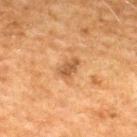size: ~3 mm (longest diameter)
location: the upper back
imaging modality: total-body-photography crop, ~15 mm field of view
patient: male, aged approximately 50
lighting: cross-polarized illumination
automated lesion analysis: an area of roughly 4 mm², a shape eccentricity near 0.85, and two-axis asymmetry of about 0.25; a lesion color around L≈45 a*≈19 b*≈34 in CIELAB and about 9 CIELAB-L* units darker than the surrounding skin; border irregularity of about 3 on a 0–10 scale and peripheral color asymmetry of about 0.5; an automated nevus-likeness rating near 0 out of 100